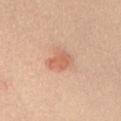A male subject in their 40s. An algorithmic analysis of the crop reported a mean CIELAB color near L≈63 a*≈26 b*≈33, a lesion–skin lightness drop of about 9, and a lesion-to-skin contrast of about 6 (normalized; higher = more distinct). And it measured border irregularity of about 2.5 on a 0–10 scale. The analysis additionally found a nevus-likeness score of about 90/100. Cropped from a whole-body photographic skin survey; the tile spans about 15 mm. The recorded lesion diameter is about 3.5 mm. The lesion is located on the upper back. This is a white-light tile.